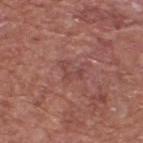  biopsy_status: not biopsied; imaged during a skin examination
  site: upper back
  image:
    source: total-body photography crop
    field_of_view_mm: 15
  patient:
    sex: male
    age_approx: 75